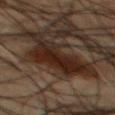<lesion>
  <biopsy_status>not biopsied; imaged during a skin examination</biopsy_status>
  <automated_metrics>
    <eccentricity>0.75</eccentricity>
    <cielab_L>17</cielab_L>
    <cielab_a>13</cielab_a>
    <cielab_b>18</cielab_b>
    <vs_skin_darker_L>8.0</vs_skin_darker_L>
    <vs_skin_contrast_norm>11.0</vs_skin_contrast_norm>
    <border_irregularity_0_10>6.0</border_irregularity_0_10>
    <color_variation_0_10>4.0</color_variation_0_10>
  </automated_metrics>
  <lesion_size>
    <long_diameter_mm_approx>7.5</long_diameter_mm_approx>
  </lesion_size>
  <image>
    <source>total-body photography crop</source>
    <field_of_view_mm>15</field_of_view_mm>
  </image>
  <patient>
    <sex>male</sex>
    <age_approx>50</age_approx>
  </patient>
  <site>mid back</site>
  <lighting>cross-polarized</lighting>
</lesion>